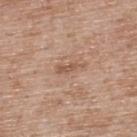notes: imaged on a skin check; not biopsied
lesion diameter: ~3.5 mm (longest diameter)
subject: male, approximately 50 years of age
imaging modality: ~15 mm tile from a whole-body skin photo
anatomic site: the upper back
TBP lesion metrics: a mean CIELAB color near L≈55 a*≈20 b*≈29 and a normalized lesion–skin contrast near 6; a border-irregularity index near 5.5/10; an automated nevus-likeness rating near 0 out of 100
lighting: white-light